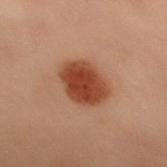Captured during whole-body skin photography for melanoma surveillance; the lesion was not biopsied. The lesion is located on the back. The patient is a female aged approximately 60. A lesion tile, about 15 mm wide, cut from a 3D total-body photograph. The recorded lesion diameter is about 4.5 mm. The total-body-photography lesion software estimated roughly 13 lightness units darker than nearby skin and a lesion-to-skin contrast of about 11 (normalized; higher = more distinct).